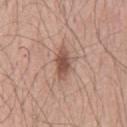Assessment: The lesion was tiled from a total-body skin photograph and was not biopsied. Image and clinical context: This image is a 15 mm lesion crop taken from a total-body photograph. A male patient, aged around 55. The lesion is on the chest.